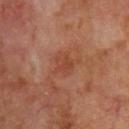A lesion tile, about 15 mm wide, cut from a 3D total-body photograph. A male subject, in their 70s. The lesion is located on the upper back. The lesion's longest dimension is about 3 mm. The tile uses cross-polarized illumination. The total-body-photography lesion software estimated an eccentricity of roughly 0.45 and a symmetry-axis asymmetry near 0.3.